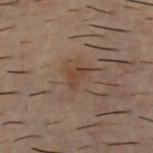Background:
A male subject about 30 years old. The tile uses cross-polarized illumination. Cropped from a total-body skin-imaging series; the visible field is about 15 mm. Measured at roughly 2.5 mm in maximum diameter. The lesion is on the chest. The lesion-visualizer software estimated a footprint of about 3.5 mm², an outline eccentricity of about 0.75 (0 = round, 1 = elongated), and two-axis asymmetry of about 0.45. The analysis additionally found border irregularity of about 4 on a 0–10 scale, a within-lesion color-variation index near 1/10, and radial color variation of about 0.5. It also reported a classifier nevus-likeness of about 5/100.